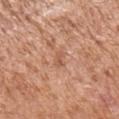A male patient, aged approximately 65. A 15 mm crop from a total-body photograph taken for skin-cancer surveillance. Longest diameter approximately 2.5 mm. The lesion is on the right upper arm.